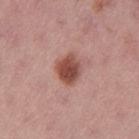workup=catalogued during a skin exam; not biopsied
site=the left thigh
size=≈3 mm
patient=female, approximately 55 years of age
TBP lesion metrics=a color-variation rating of about 3.5/10 and radial color variation of about 1; an automated nevus-likeness rating near 100 out of 100 and a detector confidence of about 100 out of 100 that the crop contains a lesion
image source=~15 mm crop, total-body skin-cancer survey